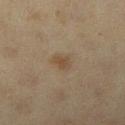Clinical impression:
No biopsy was performed on this lesion — it was imaged during a full skin examination and was not determined to be concerning.
Background:
The lesion is on the leg. A 15 mm close-up extracted from a 3D total-body photography capture. Captured under cross-polarized illumination. A female patient, roughly 40 years of age.